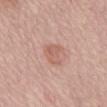No biopsy was performed on this lesion — it was imaged during a full skin examination and was not determined to be concerning. The subject is a female roughly 65 years of age. This is a white-light tile. On the mid back. The lesion's longest dimension is about 3.5 mm. A 15 mm close-up extracted from a 3D total-body photography capture. The total-body-photography lesion software estimated a lesion area of about 6 mm², an eccentricity of roughly 0.8, and two-axis asymmetry of about 0.35. The analysis additionally found a lesion color around L≈60 a*≈23 b*≈27 in CIELAB and about 8 CIELAB-L* units darker than the surrounding skin. And it measured a classifier nevus-likeness of about 25/100 and a detector confidence of about 100 out of 100 that the crop contains a lesion.